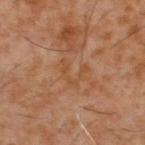* workup — catalogued during a skin exam; not biopsied
* tile lighting — cross-polarized illumination
* subject — male, in their 60s
* automated metrics — a footprint of about 5 mm² and a symmetry-axis asymmetry near 0.7; an average lesion color of about L≈45 a*≈20 b*≈33 (CIELAB), roughly 5 lightness units darker than nearby skin, and a normalized lesion–skin contrast near 5; border irregularity of about 9 on a 0–10 scale and internal color variation of about 0.5 on a 0–10 scale; an automated nevus-likeness rating near 0 out of 100 and a lesion-detection confidence of about 95/100
* image — ~15 mm crop, total-body skin-cancer survey
* diameter — ~3.5 mm (longest diameter)
* location — the upper back